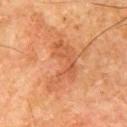workup=catalogued during a skin exam; not biopsied | patient=male, roughly 65 years of age | size=about 7.5 mm | acquisition=~15 mm crop, total-body skin-cancer survey | tile lighting=cross-polarized | image-analysis metrics=a lesion color around L≈46 a*≈23 b*≈33 in CIELAB and a lesion–skin lightness drop of about 7 | body site=the right upper arm.